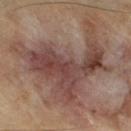Part of a total-body skin-imaging series; this lesion was reviewed on a skin check and was not flagged for biopsy. Measured at roughly 10.5 mm in maximum diameter. A male subject about 70 years old. The tile uses cross-polarized illumination. The lesion is on the leg. An algorithmic analysis of the crop reported a lesion area of about 47 mm², an eccentricity of roughly 0.6, and a symmetry-axis asymmetry near 0.65. And it measured an average lesion color of about L≈41 a*≈17 b*≈21 (CIELAB), a lesion–skin lightness drop of about 10, and a lesion-to-skin contrast of about 8.5 (normalized; higher = more distinct). The software also gave a border-irregularity index near 10/10 and radial color variation of about 1.5. It also reported an automated nevus-likeness rating near 5 out of 100 and a lesion-detection confidence of about 90/100. A region of skin cropped from a whole-body photographic capture, roughly 15 mm wide.